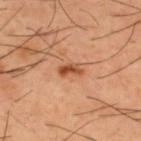The lesion was photographed on a routine skin check and not biopsied; there is no pathology result.
Imaged with cross-polarized lighting.
Longest diameter approximately 3 mm.
The lesion is located on the upper back.
A male subject aged around 40.
Automated tile analysis of the lesion measured a lesion area of about 3.5 mm², a shape eccentricity near 0.85, and a symmetry-axis asymmetry near 0.2. The software also gave a lesion color around L≈50 a*≈27 b*≈37 in CIELAB, about 13 CIELAB-L* units darker than the surrounding skin, and a normalized lesion–skin contrast near 9.
A 15 mm close-up tile from a total-body photography series done for melanoma screening.